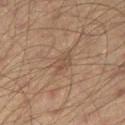A 15 mm close-up extracted from a 3D total-body photography capture. From the right thigh. A male subject approximately 45 years of age. An algorithmic analysis of the crop reported a mean CIELAB color near L≈40 a*≈14 b*≈23, roughly 5 lightness units darker than nearby skin, and a lesion-to-skin contrast of about 5 (normalized; higher = more distinct). The analysis additionally found an automated nevus-likeness rating near 0 out of 100. The tile uses cross-polarized illumination.